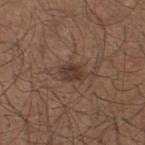Captured during whole-body skin photography for melanoma surveillance; the lesion was not biopsied.
The lesion is located on the back.
Imaged with white-light lighting.
A close-up tile cropped from a whole-body skin photograph, about 15 mm across.
A male subject, in their mid- to late 20s.